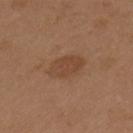No biopsy was performed on this lesion — it was imaged during a full skin examination and was not determined to be concerning. The patient is a female about 40 years old. Imaged with white-light lighting. From the right upper arm. A 15 mm crop from a total-body photograph taken for skin-cancer surveillance. Automated image analysis of the tile measured a footprint of about 8 mm² and a shape-asymmetry score of about 0.15 (0 = symmetric). It also reported a classifier nevus-likeness of about 30/100 and a lesion-detection confidence of about 100/100. The lesion's longest dimension is about 4 mm.